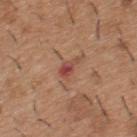{
  "biopsy_status": "not biopsied; imaged during a skin examination",
  "site": "upper back",
  "automated_metrics": {
    "area_mm2_approx": 3.0,
    "shape_asymmetry": 0.4,
    "border_irregularity_0_10": 4.5,
    "color_variation_0_10": 2.0,
    "peripheral_color_asymmetry": 1.0,
    "lesion_detection_confidence_0_100": 100
  },
  "lighting": "white-light",
  "patient": {
    "sex": "male",
    "age_approx": 40
  },
  "image": {
    "source": "total-body photography crop",
    "field_of_view_mm": 15
  },
  "lesion_size": {
    "long_diameter_mm_approx": 2.5
  }
}